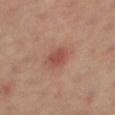Assessment: No biopsy was performed on this lesion — it was imaged during a full skin examination and was not determined to be concerning. Clinical summary: A roughly 15 mm field-of-view crop from a total-body skin photograph. A female patient aged 38 to 42. The lesion is located on the right thigh.